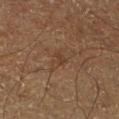Case summary:
– biopsy status: catalogued during a skin exam; not biopsied
– location: the right lower leg
– subject: male, about 65 years old
– image: 15 mm crop, total-body photography
– diameter: ~3 mm (longest diameter)
– lighting: cross-polarized